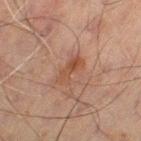The lesion was tiled from a total-body skin photograph and was not biopsied. Located on the leg. This is a cross-polarized tile. A 15 mm close-up extracted from a 3D total-body photography capture. The recorded lesion diameter is about 3.5 mm. The patient is a male aged around 60.